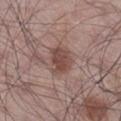The lesion was photographed on a routine skin check and not biopsied; there is no pathology result. A 15 mm crop from a total-body photograph taken for skin-cancer surveillance. The subject is a male aged around 55. The lesion is on the right lower leg.